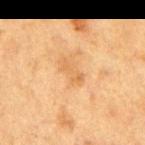Recorded during total-body skin imaging; not selected for excision or biopsy. Imaged with cross-polarized lighting. A 15 mm close-up tile from a total-body photography series done for melanoma screening. The patient is a female in their 40s. The lesion is located on the right upper arm.